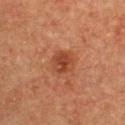This lesion was catalogued during total-body skin photography and was not selected for biopsy. A female patient aged 53 to 57. Cropped from a whole-body photographic skin survey; the tile spans about 15 mm. The recorded lesion diameter is about 2.5 mm. The tile uses cross-polarized illumination. From the upper back.